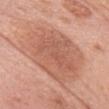Background:
Cropped from a whole-body photographic skin survey; the tile spans about 15 mm. About 9 mm across. A female patient aged around 60. An algorithmic analysis of the crop reported a footprint of about 48 mm², an outline eccentricity of about 0.7 (0 = round, 1 = elongated), and a shape-asymmetry score of about 0.2 (0 = symmetric). The analysis additionally found an average lesion color of about L≈60 a*≈24 b*≈31 (CIELAB), a lesion–skin lightness drop of about 9, and a normalized border contrast of about 6. The software also gave border irregularity of about 3 on a 0–10 scale, a color-variation rating of about 3.5/10, and peripheral color asymmetry of about 1. This is a white-light tile. From the head or neck.